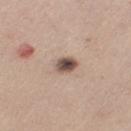notes: total-body-photography surveillance lesion; no biopsy | illumination: white-light illumination | image source: 15 mm crop, total-body photography | site: the left thigh | lesion diameter: about 2.5 mm | subject: female, aged approximately 45 | image-analysis metrics: a footprint of about 4.5 mm² and a symmetry-axis asymmetry near 0.2; about 17 CIELAB-L* units darker than the surrounding skin and a normalized border contrast of about 12.